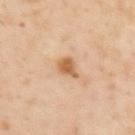Captured during whole-body skin photography for melanoma surveillance; the lesion was not biopsied. The lesion is located on the upper back. Cropped from a total-body skin-imaging series; the visible field is about 15 mm. The lesion's longest dimension is about 3 mm. A male patient, roughly 55 years of age. Captured under cross-polarized illumination.